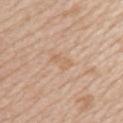notes: no biopsy performed (imaged during a skin exam)
location: the left upper arm
automated metrics: a mean CIELAB color near L≈63 a*≈18 b*≈33, roughly 5 lightness units darker than nearby skin, and a lesion-to-skin contrast of about 4.5 (normalized; higher = more distinct); border irregularity of about 4.5 on a 0–10 scale and a within-lesion color-variation index near 0/10; a detector confidence of about 100 out of 100 that the crop contains a lesion
illumination: white-light illumination
subject: female, roughly 45 years of age
size: ≈2.5 mm
imaging modality: ~15 mm tile from a whole-body skin photo